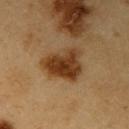Part of a total-body skin-imaging series; this lesion was reviewed on a skin check and was not flagged for biopsy.
The subject is a male roughly 60 years of age.
Imaged with cross-polarized lighting.
The lesion is on the right upper arm.
The recorded lesion diameter is about 5 mm.
This image is a 15 mm lesion crop taken from a total-body photograph.